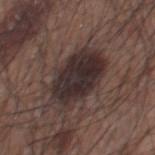<record>
  <biopsy_status>not biopsied; imaged during a skin examination</biopsy_status>
  <image>
    <source>total-body photography crop</source>
    <field_of_view_mm>15</field_of_view_mm>
  </image>
  <lighting>white-light</lighting>
  <site>chest</site>
  <lesion_size>
    <long_diameter_mm_approx>7.0</long_diameter_mm_approx>
  </lesion_size>
  <patient>
    <sex>male</sex>
    <age_approx>75</age_approx>
  </patient>
</record>